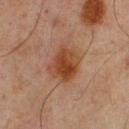Impression: This lesion was catalogued during total-body skin photography and was not selected for biopsy. Context: Located on the back. The recorded lesion diameter is about 4 mm. A roughly 15 mm field-of-view crop from a total-body skin photograph. A male patient aged around 65.